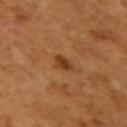Clinical impression:
Part of a total-body skin-imaging series; this lesion was reviewed on a skin check and was not flagged for biopsy.
Acquisition and patient details:
Imaged with cross-polarized lighting. A region of skin cropped from a whole-body photographic capture, roughly 15 mm wide. A female subject in their mid- to late 50s. The lesion is on the right upper arm.